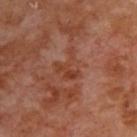Recorded during total-body skin imaging; not selected for excision or biopsy.
A 15 mm crop from a total-body photograph taken for skin-cancer surveillance.
The lesion is on the back.
The total-body-photography lesion software estimated a lesion area of about 5.5 mm², an outline eccentricity of about 0.65 (0 = round, 1 = elongated), and two-axis asymmetry of about 0.6. It also reported a mean CIELAB color near L≈40 a*≈25 b*≈30, about 6 CIELAB-L* units darker than the surrounding skin, and a normalized lesion–skin contrast near 6. And it measured a color-variation rating of about 2.5/10 and peripheral color asymmetry of about 0.5. And it measured an automated nevus-likeness rating near 0 out of 100 and a detector confidence of about 100 out of 100 that the crop contains a lesion.
The recorded lesion diameter is about 3.5 mm.
The subject is a male aged 68 to 72.